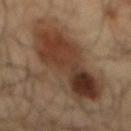Automated tile analysis of the lesion measured an area of roughly 48 mm² and a shape eccentricity near 0.9. The analysis additionally found a nevus-likeness score of about 65/100 and lesion-presence confidence of about 100/100. A 15 mm close-up tile from a total-body photography series done for melanoma screening. A male subject in their mid- to late 60s. The lesion is on the back. The recorded lesion diameter is about 12.5 mm.